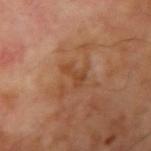  image:
    source: total-body photography crop
    field_of_view_mm: 15
  patient:
    sex: male
    age_approx: 70
  site: right upper arm
  lighting: cross-polarized